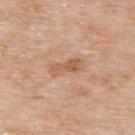notes: no biopsy performed (imaged during a skin exam) | imaging modality: 15 mm crop, total-body photography | subject: male, aged approximately 75 | automated metrics: a lesion area of about 5.5 mm², a shape eccentricity near 0.9, and a shape-asymmetry score of about 0.2 (0 = symmetric); a mean CIELAB color near L≈59 a*≈22 b*≈34, a lesion–skin lightness drop of about 9, and a normalized border contrast of about 6.5; a border-irregularity index near 3/10, internal color variation of about 3 on a 0–10 scale, and a peripheral color-asymmetry measure near 1; a nevus-likeness score of about 0/100 and a lesion-detection confidence of about 100/100 | illumination: white-light illumination | anatomic site: the upper back.